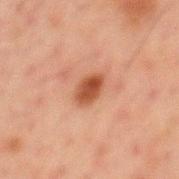Impression: No biopsy was performed on this lesion — it was imaged during a full skin examination and was not determined to be concerning. Image and clinical context: A close-up tile cropped from a whole-body skin photograph, about 15 mm across. On the back. Captured under cross-polarized illumination. About 3.5 mm across. A male patient aged 48–52.